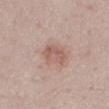workup — catalogued during a skin exam; not biopsied | lesion size — ~3.5 mm (longest diameter) | imaging modality — 15 mm crop, total-body photography | site — the mid back | subject — female, in their 50s.